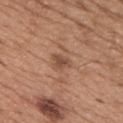Impression: Part of a total-body skin-imaging series; this lesion was reviewed on a skin check and was not flagged for biopsy. Background: Captured under white-light illumination. A region of skin cropped from a whole-body photographic capture, roughly 15 mm wide. Located on the chest. Approximately 3 mm at its widest. A male patient in their mid-60s.